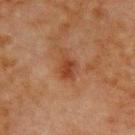A roughly 15 mm field-of-view crop from a total-body skin photograph.
The tile uses cross-polarized illumination.
The lesion is located on the chest.
A male subject roughly 70 years of age.
Measured at roughly 3 mm in maximum diameter.